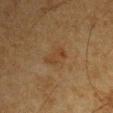Assessment:
No biopsy was performed on this lesion — it was imaged during a full skin examination and was not determined to be concerning.
Context:
An algorithmic analysis of the crop reported an outline eccentricity of about 0.65 (0 = round, 1 = elongated) and a shape-asymmetry score of about 0.3 (0 = symmetric). It also reported border irregularity of about 3.5 on a 0–10 scale, a within-lesion color-variation index near 3/10, and peripheral color asymmetry of about 1. It also reported a nevus-likeness score of about 0/100. On the left upper arm. The patient is a male in their mid-60s. A 15 mm close-up extracted from a 3D total-body photography capture. Imaged with cross-polarized lighting.